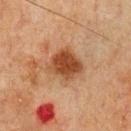Part of a total-body skin-imaging series; this lesion was reviewed on a skin check and was not flagged for biopsy. A 15 mm close-up tile from a total-body photography series done for melanoma screening. Imaged with cross-polarized lighting. A male subject, aged 63 to 67. On the chest.